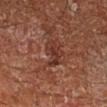Assessment:
This lesion was catalogued during total-body skin photography and was not selected for biopsy.
Clinical summary:
A male subject, aged around 65. Automated image analysis of the tile measured a shape eccentricity near 0.7 and two-axis asymmetry of about 0.5. And it measured an average lesion color of about L≈33 a*≈23 b*≈26 (CIELAB), about 7 CIELAB-L* units darker than the surrounding skin, and a normalized lesion–skin contrast near 7. The analysis additionally found border irregularity of about 5.5 on a 0–10 scale and peripheral color asymmetry of about 1. The lesion is on the right lower leg. A 15 mm close-up tile from a total-body photography series done for melanoma screening. This is a cross-polarized tile. Measured at roughly 3.5 mm in maximum diameter.